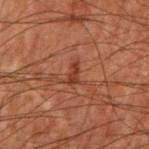Findings:
• workup · no biopsy performed (imaged during a skin exam)
• imaging modality · ~15 mm crop, total-body skin-cancer survey
• patient · male, in their 70s
• body site · the right forearm
• automated lesion analysis · a footprint of about 3 mm²; a border-irregularity rating of about 3.5/10, internal color variation of about 1 on a 0–10 scale, and radial color variation of about 0.5; a classifier nevus-likeness of about 0/100